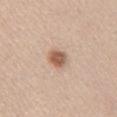Image and clinical context: About 3 mm across. The tile uses white-light illumination. A lesion tile, about 15 mm wide, cut from a 3D total-body photograph. The total-body-photography lesion software estimated an average lesion color of about L≈59 a*≈19 b*≈30 (CIELAB), roughly 14 lightness units darker than nearby skin, and a normalized border contrast of about 9. And it measured a lesion-detection confidence of about 100/100. On the left upper arm. The subject is a female aged 38–42.